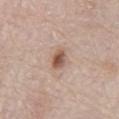| feature | finding |
|---|---|
| workup | catalogued during a skin exam; not biopsied |
| acquisition | ~15 mm tile from a whole-body skin photo |
| tile lighting | white-light |
| location | the abdomen |
| automated lesion analysis | a border-irregularity rating of about 1.5/10, a within-lesion color-variation index near 4.5/10, and peripheral color asymmetry of about 1.5 |
| subject | male, aged 78–82 |
| lesion size | ~2.5 mm (longest diameter) |